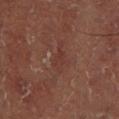| field | value |
|---|---|
| follow-up | catalogued during a skin exam; not biopsied |
| lighting | cross-polarized |
| image | total-body-photography crop, ~15 mm field of view |
| anatomic site | the right lower leg |
| automated lesion analysis | a footprint of about 3.5 mm² and a shape-asymmetry score of about 0.45 (0 = symmetric); a mean CIELAB color near L≈31 a*≈20 b*≈21 and a lesion-to-skin contrast of about 5 (normalized; higher = more distinct); a within-lesion color-variation index near 1/10 and radial color variation of about 0.5; a classifier nevus-likeness of about 0/100 |
| subject | male, aged 48–52 |